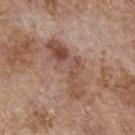No biopsy was performed on this lesion — it was imaged during a full skin examination and was not determined to be concerning.